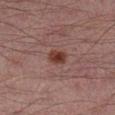No biopsy was performed on this lesion — it was imaged during a full skin examination and was not determined to be concerning. Measured at roughly 2.5 mm in maximum diameter. On the right lower leg. Automated image analysis of the tile measured a lesion area of about 4.5 mm² and an eccentricity of roughly 0.6. The analysis additionally found a nevus-likeness score of about 95/100. Cropped from a total-body skin-imaging series; the visible field is about 15 mm. A male patient aged approximately 30.